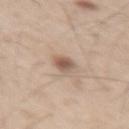Context:
The patient is a male about 60 years old. Measured at roughly 2.5 mm in maximum diameter. The lesion is on the right thigh. Captured under white-light illumination. A roughly 15 mm field-of-view crop from a total-body skin photograph.